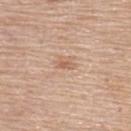* follow-up · imaged on a skin check; not biopsied
* image-analysis metrics · an area of roughly 2 mm² and two-axis asymmetry of about 0.3; an automated nevus-likeness rating near 0 out of 100 and lesion-presence confidence of about 100/100
* lighting · white-light illumination
* lesion size · about 2 mm
* patient · female, aged 63 to 67
* body site · the upper back
* imaging modality · 15 mm crop, total-body photography